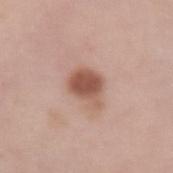No biopsy was performed on this lesion — it was imaged during a full skin examination and was not determined to be concerning. The lesion-visualizer software estimated an area of roughly 9.5 mm² and an outline eccentricity of about 0.65 (0 = round, 1 = elongated). The analysis additionally found about 13 CIELAB-L* units darker than the surrounding skin and a normalized border contrast of about 9. The tile uses white-light illumination. This image is a 15 mm lesion crop taken from a total-body photograph. The recorded lesion diameter is about 4 mm. A female subject aged 48–52. Located on the left forearm.